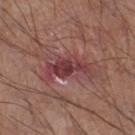The lesion was photographed on a routine skin check and not biopsied; there is no pathology result.
On the left lower leg.
The tile uses white-light illumination.
Approximately 5 mm at its widest.
This image is a 15 mm lesion crop taken from a total-body photograph.
The subject is a male approximately 70 years of age.